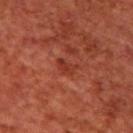Assessment:
No biopsy was performed on this lesion — it was imaged during a full skin examination and was not determined to be concerning.
Image and clinical context:
A 15 mm crop from a total-body photograph taken for skin-cancer surveillance. A male patient, approximately 70 years of age. The lesion is on the back.